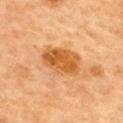<case>
<biopsy_status>not biopsied; imaged during a skin examination</biopsy_status>
</case>